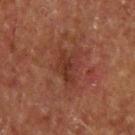follow-up = catalogued during a skin exam; not biopsied | size = about 4 mm | anatomic site = the upper back | imaging modality = ~15 mm tile from a whole-body skin photo | TBP lesion metrics = a footprint of about 6.5 mm², an eccentricity of roughly 0.8, and two-axis asymmetry of about 0.3; a border-irregularity rating of about 4.5/10 and radial color variation of about 1; a classifier nevus-likeness of about 0/100 and lesion-presence confidence of about 100/100 | patient = male, aged around 55.